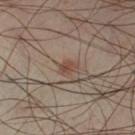{
  "biopsy_status": "not biopsied; imaged during a skin examination"
}